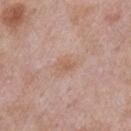notes = catalogued during a skin exam; not biopsied
imaging modality = total-body-photography crop, ~15 mm field of view
site = the right upper arm
TBP lesion metrics = a lesion area of about 3.5 mm² and a shape eccentricity near 0.75; border irregularity of about 2.5 on a 0–10 scale
illumination = white-light illumination
size = about 2.5 mm
subject = male, in their mid-50s